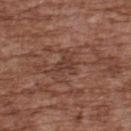{
  "image": {
    "source": "total-body photography crop",
    "field_of_view_mm": 15
  },
  "automated_metrics": {
    "area_mm2_approx": 4.0,
    "eccentricity": 0.6,
    "cielab_L": 39,
    "cielab_a": 20,
    "cielab_b": 25,
    "vs_skin_darker_L": 7.0,
    "border_irregularity_0_10": 4.5,
    "color_variation_0_10": 1.5,
    "peripheral_color_asymmetry": 0.5,
    "nevus_likeness_0_100": 0,
    "lesion_detection_confidence_0_100": 70
  },
  "patient": {
    "sex": "male",
    "age_approx": 75
  },
  "lesion_size": {
    "long_diameter_mm_approx": 2.5
  },
  "lighting": "white-light",
  "site": "upper back"
}